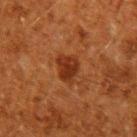{"biopsy_status": "not biopsied; imaged during a skin examination", "image": {"source": "total-body photography crop", "field_of_view_mm": 15}, "lighting": "cross-polarized", "site": "right upper arm", "patient": {"sex": "male", "age_approx": 60}, "automated_metrics": {"nevus_likeness_0_100": 85}, "lesion_size": {"long_diameter_mm_approx": 3.0}}